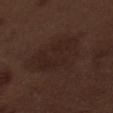| field | value |
|---|---|
| workup | no biopsy performed (imaged during a skin exam) |
| location | the front of the torso |
| size | ~8 mm (longest diameter) |
| tile lighting | white-light illumination |
| subject | male, about 70 years old |
| automated metrics | a footprint of about 25 mm², a shape eccentricity near 0.8, and two-axis asymmetry of about 0.35; an average lesion color of about L≈22 a*≈15 b*≈19 (CIELAB), a lesion–skin lightness drop of about 5, and a normalized lesion–skin contrast near 6; a border-irregularity index near 4/10 and internal color variation of about 2.5 on a 0–10 scale; a nevus-likeness score of about 0/100 and a detector confidence of about 100 out of 100 that the crop contains a lesion |
| image source | ~15 mm crop, total-body skin-cancer survey |